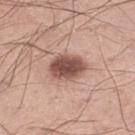Imaged during a routine full-body skin examination; the lesion was not biopsied and no histopathology is available.
A 15 mm close-up extracted from a 3D total-body photography capture.
Approximately 4.5 mm at its widest.
A male subject, roughly 40 years of age.
The total-body-photography lesion software estimated a lesion area of about 11 mm² and two-axis asymmetry of about 0.15.
This is a white-light tile.
The lesion is on the left lower leg.